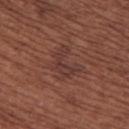Findings:
- workup: total-body-photography surveillance lesion; no biopsy
- location: the upper back
- illumination: white-light
- diameter: ≈4 mm
- subject: female, aged 63–67
- acquisition: 15 mm crop, total-body photography